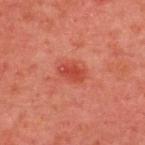Clinical impression:
Recorded during total-body skin imaging; not selected for excision or biopsy.
Acquisition and patient details:
On the upper back. Imaged with cross-polarized lighting. The recorded lesion diameter is about 3 mm. This image is a 15 mm lesion crop taken from a total-body photograph. A male patient aged 43 to 47.